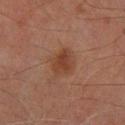Assessment: The lesion was tiled from a total-body skin photograph and was not biopsied. Image and clinical context: A male patient, roughly 70 years of age. The tile uses cross-polarized illumination. Approximately 3 mm at its widest. A close-up tile cropped from a whole-body skin photograph, about 15 mm across. From the left lower leg.